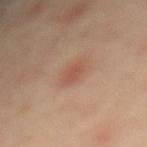Impression:
Imaged during a routine full-body skin examination; the lesion was not biopsied and no histopathology is available.
Clinical summary:
This is a cross-polarized tile. A female subject aged approximately 40. From the mid back. A close-up tile cropped from a whole-body skin photograph, about 15 mm across. Longest diameter approximately 2.5 mm.